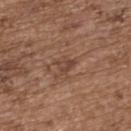<tbp_lesion>
<biopsy_status>not biopsied; imaged during a skin examination</biopsy_status>
<lighting>white-light</lighting>
<image>
  <source>total-body photography crop</source>
  <field_of_view_mm>15</field_of_view_mm>
</image>
<automated_metrics>
  <area_mm2_approx>4.0</area_mm2_approx>
  <eccentricity>0.6</eccentricity>
  <shape_asymmetry>0.45</shape_asymmetry>
  <vs_skin_darker_L>7.0</vs_skin_darker_L>
</automated_metrics>
<patient>
  <sex>female</sex>
  <age_approx>65</age_approx>
</patient>
<site>upper back</site>
<lesion_size>
  <long_diameter_mm_approx>2.5</long_diameter_mm_approx>
</lesion_size>
</tbp_lesion>